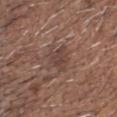Cropped from a whole-body photographic skin survey; the tile spans about 15 mm. A male patient, roughly 65 years of age. Approximately 4 mm at its widest. On the head or neck. The lesion-visualizer software estimated a shape eccentricity near 0.8 and two-axis asymmetry of about 0.3. And it measured a mean CIELAB color near L≈42 a*≈17 b*≈22 and roughly 8 lightness units darker than nearby skin. The software also gave border irregularity of about 3.5 on a 0–10 scale, internal color variation of about 2.5 on a 0–10 scale, and radial color variation of about 1. The analysis additionally found a classifier nevus-likeness of about 0/100 and a lesion-detection confidence of about 95/100.